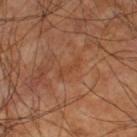Clinical impression: Captured during whole-body skin photography for melanoma surveillance; the lesion was not biopsied. Image and clinical context: Measured at roughly 3.5 mm in maximum diameter. The patient is a male aged approximately 60. This is a cross-polarized tile. On the left thigh. A 15 mm close-up tile from a total-body photography series done for melanoma screening.